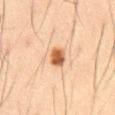Assessment: Imaged during a routine full-body skin examination; the lesion was not biopsied and no histopathology is available. Background: Automated tile analysis of the lesion measured a footprint of about 4.5 mm², an eccentricity of roughly 0.65, and two-axis asymmetry of about 0.15. And it measured a lesion color around L≈49 a*≈21 b*≈33 in CIELAB and a normalized border contrast of about 11. The recorded lesion diameter is about 2.5 mm. Cropped from a total-body skin-imaging series; the visible field is about 15 mm. A male patient aged approximately 60. Captured under cross-polarized illumination. Located on the front of the torso.